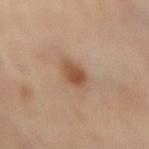Q: Was a biopsy performed?
A: total-body-photography surveillance lesion; no biopsy
Q: Patient demographics?
A: male, aged around 65
Q: How large is the lesion?
A: ~2.5 mm (longest diameter)
Q: Illumination type?
A: cross-polarized illumination
Q: Lesion location?
A: the lower back
Q: What kind of image is this?
A: ~15 mm crop, total-body skin-cancer survey